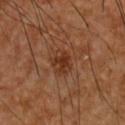Impression: The lesion was photographed on a routine skin check and not biopsied; there is no pathology result. Acquisition and patient details: Cropped from a whole-body photographic skin survey; the tile spans about 15 mm. The lesion is located on the chest. Approximately 3 mm at its widest. Captured under cross-polarized illumination. A male patient in their mid- to late 40s.